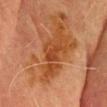Clinical impression: Imaged during a routine full-body skin examination; the lesion was not biopsied and no histopathology is available.